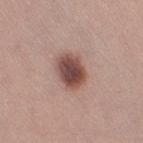Clinical impression: This lesion was catalogued during total-body skin photography and was not selected for biopsy. Clinical summary: Measured at roughly 4 mm in maximum diameter. This image is a 15 mm lesion crop taken from a total-body photograph. From the right thigh. A female subject aged approximately 35. Imaged with white-light lighting. The lesion-visualizer software estimated an average lesion color of about L≈48 a*≈20 b*≈23 (CIELAB) and a normalized border contrast of about 11. The analysis additionally found border irregularity of about 1.5 on a 0–10 scale, internal color variation of about 5.5 on a 0–10 scale, and peripheral color asymmetry of about 1.5. The analysis additionally found a classifier nevus-likeness of about 95/100 and a lesion-detection confidence of about 100/100.